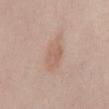Case summary:
* site · the mid back
* acquisition · ~15 mm crop, total-body skin-cancer survey
* lesion size · about 4.5 mm
* patient · male, in their mid-20s
* TBP lesion metrics · a lesion area of about 8.5 mm² and a symmetry-axis asymmetry near 0.2; a border-irregularity index near 2.5/10, internal color variation of about 2 on a 0–10 scale, and a peripheral color-asymmetry measure near 0.5
* lighting · white-light illumination